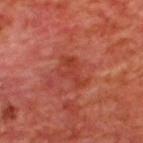Impression: Recorded during total-body skin imaging; not selected for excision or biopsy. Context: A region of skin cropped from a whole-body photographic capture, roughly 15 mm wide. Captured under cross-polarized illumination. A male subject, aged around 65. On the upper back.